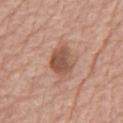Impression:
This lesion was catalogued during total-body skin photography and was not selected for biopsy.
Acquisition and patient details:
The total-body-photography lesion software estimated a mean CIELAB color near L≈52 a*≈21 b*≈29 and a lesion–skin lightness drop of about 12. And it measured border irregularity of about 2.5 on a 0–10 scale and internal color variation of about 2.5 on a 0–10 scale. A 15 mm close-up tile from a total-body photography series done for melanoma screening. This is a white-light tile. Located on the chest. The patient is a male about 75 years old. About 3.5 mm across.